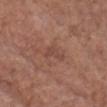Clinical impression: Recorded during total-body skin imaging; not selected for excision or biopsy. Clinical summary: A male subject, aged 78 to 82. From the chest. A 15 mm close-up extracted from a 3D total-body photography capture.